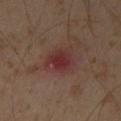Impression:
Part of a total-body skin-imaging series; this lesion was reviewed on a skin check and was not flagged for biopsy.
Acquisition and patient details:
A 15 mm close-up tile from a total-body photography series done for melanoma screening. The subject is a male roughly 60 years of age. Captured under cross-polarized illumination. The lesion is located on the left thigh.